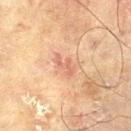{"biopsy_status": "not biopsied; imaged during a skin examination", "site": "left thigh", "image": {"source": "total-body photography crop", "field_of_view_mm": 15}, "lighting": "cross-polarized", "patient": {"sex": "male", "age_approx": 70}, "automated_metrics": {"nevus_likeness_0_100": 0, "lesion_detection_confidence_0_100": 100}}